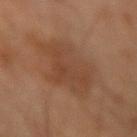The lesion was tiled from a total-body skin photograph and was not biopsied. Imaged with cross-polarized lighting. The lesion is located on the right forearm. The total-body-photography lesion software estimated a border-irregularity index near 4.5/10 and a peripheral color-asymmetry measure near 1. A male patient aged 68–72. A roughly 15 mm field-of-view crop from a total-body skin photograph. The recorded lesion diameter is about 6.5 mm.